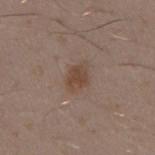The lesion was photographed on a routine skin check and not biopsied; there is no pathology result.
This image is a 15 mm lesion crop taken from a total-body photograph.
A male subject aged 28–32.
From the right upper arm.